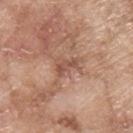Impression: No biopsy was performed on this lesion — it was imaged during a full skin examination and was not determined to be concerning. Background: The total-body-photography lesion software estimated roughly 9 lightness units darker than nearby skin and a normalized border contrast of about 6.5. This is a white-light tile. A 15 mm close-up tile from a total-body photography series done for melanoma screening. A male patient, aged 63–67. The lesion is located on the upper back.